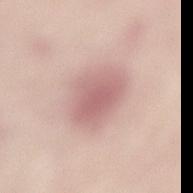biopsy status: imaged on a skin check; not biopsied
TBP lesion metrics: a lesion color around L≈64 a*≈21 b*≈22 in CIELAB, roughly 10 lightness units darker than nearby skin, and a normalized border contrast of about 6.5; a nevus-likeness score of about 10/100
image source: total-body-photography crop, ~15 mm field of view
patient: female, aged around 30
illumination: white-light
lesion diameter: ≈5 mm
location: the lower back